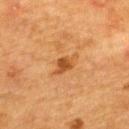workup = catalogued during a skin exam; not biopsied
lighting = cross-polarized
imaging modality = 15 mm crop, total-body photography
automated metrics = a mean CIELAB color near L≈43 a*≈22 b*≈37 and a normalized lesion–skin contrast near 7.5; a border-irregularity rating of about 3.5/10 and a color-variation rating of about 3/10; a nevus-likeness score of about 60/100 and a lesion-detection confidence of about 100/100
subject = female, aged around 55
anatomic site = the upper back
lesion size = about 3.5 mm